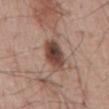Assessment:
The lesion was photographed on a routine skin check and not biopsied; there is no pathology result.
Context:
Cropped from a total-body skin-imaging series; the visible field is about 15 mm. Approximately 4.5 mm at its widest. On the abdomen. Imaged with white-light lighting. A male subject, roughly 65 years of age.